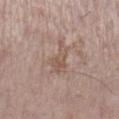follow-up: imaged on a skin check; not biopsied
subject: female, roughly 65 years of age
site: the right lower leg
size: ~4.5 mm (longest diameter)
imaging modality: total-body-photography crop, ~15 mm field of view